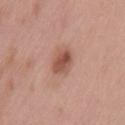Q: Is there a histopathology result?
A: no biopsy performed (imaged during a skin exam)
Q: What kind of image is this?
A: 15 mm crop, total-body photography
Q: What lighting was used for the tile?
A: white-light
Q: What are the patient's age and sex?
A: male, aged 53–57
Q: Where on the body is the lesion?
A: the lower back